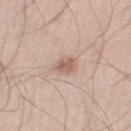Q: Was this lesion biopsied?
A: no biopsy performed (imaged during a skin exam)
Q: Lesion location?
A: the leg
Q: Automated lesion metrics?
A: a border-irregularity index near 2/10, a color-variation rating of about 2.5/10, and radial color variation of about 1; a nevus-likeness score of about 25/100 and lesion-presence confidence of about 100/100
Q: What is the imaging modality?
A: total-body-photography crop, ~15 mm field of view
Q: Lesion size?
A: ≈3 mm
Q: What lighting was used for the tile?
A: white-light
Q: Who is the patient?
A: male, aged 23 to 27